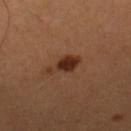<case>
<biopsy_status>not biopsied; imaged during a skin examination</biopsy_status>
<lighting>cross-polarized</lighting>
<lesion_size>
  <long_diameter_mm_approx>4.0</long_diameter_mm_approx>
</lesion_size>
<patient>
  <sex>female</sex>
  <age_approx>55</age_approx>
</patient>
<image>
  <source>total-body photography crop</source>
  <field_of_view_mm>15</field_of_view_mm>
</image>
<site>leg</site>
</case>